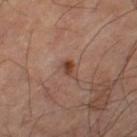– biopsy status: catalogued during a skin exam; not biopsied
– body site: the left thigh
– image: ~15 mm crop, total-body skin-cancer survey
– patient: male, aged 63 to 67